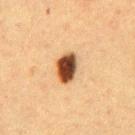This lesion was catalogued during total-body skin photography and was not selected for biopsy. The patient is a male approximately 50 years of age. The lesion is located on the abdomen. A 15 mm crop from a total-body photograph taken for skin-cancer surveillance. Automated image analysis of the tile measured a lesion area of about 7 mm² and a shape eccentricity near 0.75. It also reported a mean CIELAB color near L≈37 a*≈20 b*≈30, roughly 22 lightness units darker than nearby skin, and a normalized border contrast of about 16.5. The tile uses cross-polarized illumination. About 3.5 mm across.